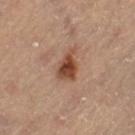The lesion was tiled from a total-body skin photograph and was not biopsied. The patient is a female aged 63–67. On the left thigh. Longest diameter approximately 4 mm. Cropped from a whole-body photographic skin survey; the tile spans about 15 mm. This is a cross-polarized tile.